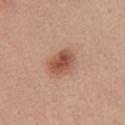workup: catalogued during a skin exam; not biopsied
anatomic site: the chest
subject: female, about 40 years old
imaging modality: total-body-photography crop, ~15 mm field of view